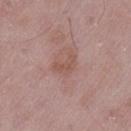This lesion was catalogued during total-body skin photography and was not selected for biopsy. The tile uses white-light illumination. On the left thigh. A male patient aged 48–52. A 15 mm close-up extracted from a 3D total-body photography capture. The lesion's longest dimension is about 3 mm.